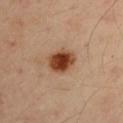– workup · total-body-photography surveillance lesion; no biopsy
– subject · male, aged 48–52
– image source · ~15 mm crop, total-body skin-cancer survey
– anatomic site · the left upper arm
– diameter · ≈3.5 mm